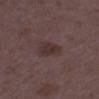Notes:
- biopsy status — catalogued during a skin exam; not biopsied
- patient — female, in their mid- to late 30s
- acquisition — ~15 mm tile from a whole-body skin photo
- size — ~3.5 mm (longest diameter)
- location — the right thigh